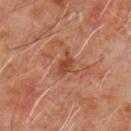Recorded during total-body skin imaging; not selected for excision or biopsy. Imaged with cross-polarized lighting. A male patient, approximately 60 years of age. A close-up tile cropped from a whole-body skin photograph, about 15 mm across. On the chest. The lesion's longest dimension is about 3 mm.